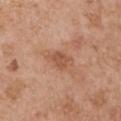  biopsy_status: not biopsied; imaged during a skin examination
  site: left upper arm
  image:
    source: total-body photography crop
    field_of_view_mm: 15
  lesion_size:
    long_diameter_mm_approx: 3.0
  automated_metrics:
    area_mm2_approx: 5.5
    shape_asymmetry: 0.35
    cielab_L: 54
    cielab_a: 23
    cielab_b: 32
    vs_skin_contrast_norm: 6.0
  patient:
    sex: male
    age_approx: 55
  lighting: white-light